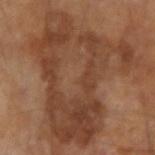Imaged during a routine full-body skin examination; the lesion was not biopsied and no histopathology is available. Approximately 14.5 mm at its widest. From the left arm. A male patient, aged around 65. A lesion tile, about 15 mm wide, cut from a 3D total-body photograph. Captured under cross-polarized illumination.